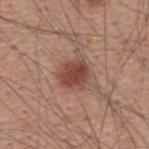Part of a total-body skin-imaging series; this lesion was reviewed on a skin check and was not flagged for biopsy. Imaged with white-light lighting. From the back. The patient is a male roughly 60 years of age. A close-up tile cropped from a whole-body skin photograph, about 15 mm across.